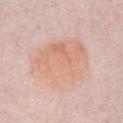Measured at roughly 7.5 mm in maximum diameter.
On the abdomen.
This is a white-light tile.
A roughly 15 mm field-of-view crop from a total-body skin photograph.
A female patient in their 60s.